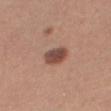Impression:
Captured during whole-body skin photography for melanoma surveillance; the lesion was not biopsied.
Context:
A female patient in their mid-30s. The lesion is located on the right thigh. This is a white-light tile. Approximately 3 mm at its widest. A 15 mm crop from a total-body photograph taken for skin-cancer surveillance. The total-body-photography lesion software estimated a footprint of about 6.5 mm², a shape eccentricity near 0.75, and a shape-asymmetry score of about 0.2 (0 = symmetric). It also reported an average lesion color of about L≈48 a*≈21 b*≈26 (CIELAB) and a normalized lesion–skin contrast near 9.5. The analysis additionally found a border-irregularity rating of about 2/10, a within-lesion color-variation index near 4.5/10, and peripheral color asymmetry of about 1.5. The software also gave a classifier nevus-likeness of about 90/100 and a detector confidence of about 100 out of 100 that the crop contains a lesion.